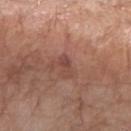Findings:
- workup · catalogued during a skin exam; not biopsied
- subject · female, aged 73 to 77
- tile lighting · white-light
- lesion diameter · about 2.5 mm
- image · ~15 mm tile from a whole-body skin photo
- TBP lesion metrics · two-axis asymmetry of about 0.4
- anatomic site · the left forearm